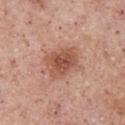Clinical impression:
Imaged during a routine full-body skin examination; the lesion was not biopsied and no histopathology is available.
Image and clinical context:
The patient is a male roughly 55 years of age. From the chest. Imaged with white-light lighting. Cropped from a whole-body photographic skin survey; the tile spans about 15 mm.